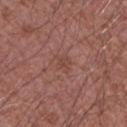Automated tile analysis of the lesion measured a lesion color around L≈45 a*≈22 b*≈25 in CIELAB and a normalized border contrast of about 4.5. It also reported a classifier nevus-likeness of about 0/100 and lesion-presence confidence of about 100/100. The recorded lesion diameter is about 2.5 mm. From the left upper arm. A roughly 15 mm field-of-view crop from a total-body skin photograph. A male subject, aged 48–52. Captured under white-light illumination.